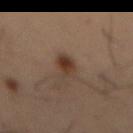  biopsy_status: not biopsied; imaged during a skin examination
  patient:
    sex: male
    age_approx: 55
  lighting: cross-polarized
  site: abdomen
  image:
    source: total-body photography crop
    field_of_view_mm: 15